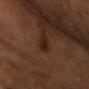| feature | finding |
|---|---|
| workup | catalogued during a skin exam; not biopsied |
| patient | male, in their 40s |
| tile lighting | cross-polarized |
| anatomic site | the chest |
| acquisition | total-body-photography crop, ~15 mm field of view |